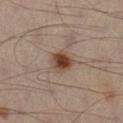biopsy status=total-body-photography surveillance lesion; no biopsy
diameter=≈3 mm
patient=male, aged 48 to 52
image source=15 mm crop, total-body photography
illumination=cross-polarized
body site=the leg